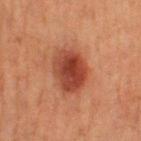Impression: The lesion was tiled from a total-body skin photograph and was not biopsied. Context: Automated image analysis of the tile measured a border-irregularity rating of about 1.5/10 and peripheral color asymmetry of about 1.5. And it measured a classifier nevus-likeness of about 100/100 and a detector confidence of about 100 out of 100 that the crop contains a lesion. On the right thigh. Longest diameter approximately 5 mm. A 15 mm close-up tile from a total-body photography series done for melanoma screening. The patient is a female aged approximately 40. Captured under cross-polarized illumination.